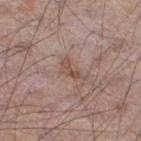Q: Was a biopsy performed?
A: imaged on a skin check; not biopsied
Q: What are the patient's age and sex?
A: male, roughly 55 years of age
Q: How was this image acquired?
A: ~15 mm crop, total-body skin-cancer survey
Q: Lesion location?
A: the right thigh
Q: Lesion size?
A: about 3.5 mm